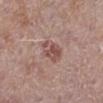Acquisition and patient details: A close-up tile cropped from a whole-body skin photograph, about 15 mm across. The patient is a male in their 80s. From the left leg.